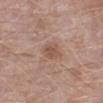Part of a total-body skin-imaging series; this lesion was reviewed on a skin check and was not flagged for biopsy.
The recorded lesion diameter is about 2.5 mm.
Automated image analysis of the tile measured an area of roughly 4 mm², an eccentricity of roughly 0.65, and a shape-asymmetry score of about 0.25 (0 = symmetric). And it measured a color-variation rating of about 2/10.
A male subject aged 48–52.
A 15 mm close-up extracted from a 3D total-body photography capture.
Located on the left lower leg.